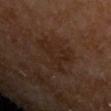Clinical impression: No biopsy was performed on this lesion — it was imaged during a full skin examination and was not determined to be concerning. Clinical summary: Measured at roughly 5 mm in maximum diameter. The lesion is located on the left upper arm. The total-body-photography lesion software estimated a footprint of about 10 mm², an outline eccentricity of about 0.7 (0 = round, 1 = elongated), and a symmetry-axis asymmetry near 0.6. A male patient roughly 65 years of age. A close-up tile cropped from a whole-body skin photograph, about 15 mm across.